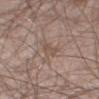Clinical impression: Captured during whole-body skin photography for melanoma surveillance; the lesion was not biopsied. Clinical summary: Located on the right thigh. Longest diameter approximately 2.5 mm. The tile uses white-light illumination. Cropped from a total-body skin-imaging series; the visible field is about 15 mm. Automated tile analysis of the lesion measured an eccentricity of roughly 0.75 and a symmetry-axis asymmetry near 0.3. The software also gave a normalized border contrast of about 5. The analysis additionally found lesion-presence confidence of about 85/100. A male subject about 65 years old.